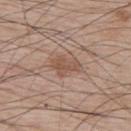body site: the back; acquisition: ~15 mm crop, total-body skin-cancer survey; subject: male, aged approximately 65.